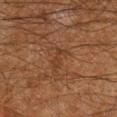Impression: Imaged during a routine full-body skin examination; the lesion was not biopsied and no histopathology is available. Context: The lesion's longest dimension is about 3 mm. A 15 mm crop from a total-body photograph taken for skin-cancer surveillance. Automated tile analysis of the lesion measured an automated nevus-likeness rating near 0 out of 100 and lesion-presence confidence of about 95/100. On the right lower leg. The tile uses cross-polarized illumination. A male subject, aged approximately 60.